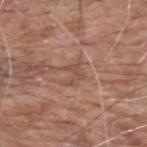Q: Is there a histopathology result?
A: total-body-photography surveillance lesion; no biopsy
Q: Where on the body is the lesion?
A: the upper back
Q: Who is the patient?
A: male, aged 58 to 62
Q: What is the imaging modality?
A: 15 mm crop, total-body photography